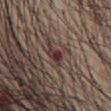Clinical impression:
The lesion was tiled from a total-body skin photograph and was not biopsied.